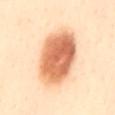This lesion was catalogued during total-body skin photography and was not selected for biopsy.
A female patient, aged approximately 40.
Longest diameter approximately 8 mm.
On the back.
A 15 mm close-up extracted from a 3D total-body photography capture.